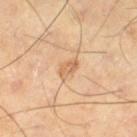Captured during whole-body skin photography for melanoma surveillance; the lesion was not biopsied. The lesion-visualizer software estimated an area of roughly 4 mm², a shape eccentricity near 0.8, and two-axis asymmetry of about 0.2. It also reported a lesion color around L≈63 a*≈20 b*≈37 in CIELAB and about 9 CIELAB-L* units darker than the surrounding skin. The tile uses cross-polarized illumination. A male patient, aged 68–72. A region of skin cropped from a whole-body photographic capture, roughly 15 mm wide. From the right thigh. The recorded lesion diameter is about 2.5 mm.